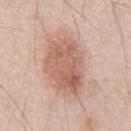Context: Automated image analysis of the tile measured a lesion area of about 26 mm², a shape eccentricity near 0.85, and two-axis asymmetry of about 0.15. The analysis additionally found a border-irregularity index near 2.5/10, a color-variation rating of about 5/10, and a peripheral color-asymmetry measure near 2. A male patient aged 43–47. A roughly 15 mm field-of-view crop from a total-body skin photograph. The tile uses white-light illumination. Longest diameter approximately 7.5 mm. The lesion is located on the chest.